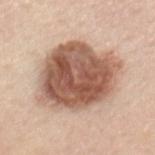This lesion was catalogued during total-body skin photography and was not selected for biopsy. A female subject about 45 years old. An algorithmic analysis of the crop reported a mean CIELAB color near L≈56 a*≈21 b*≈29 and about 18 CIELAB-L* units darker than the surrounding skin. And it measured a border-irregularity index near 2/10, a color-variation rating of about 6.5/10, and radial color variation of about 2. And it measured an automated nevus-likeness rating near 90 out of 100 and a detector confidence of about 100 out of 100 that the crop contains a lesion. Measured at roughly 9 mm in maximum diameter. A close-up tile cropped from a whole-body skin photograph, about 15 mm across. The lesion is on the mid back. Imaged with white-light lighting.